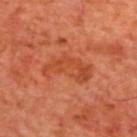Q: Is there a histopathology result?
A: imaged on a skin check; not biopsied
Q: What did automated image analysis measure?
A: an area of roughly 12 mm², a shape eccentricity near 0.9, and a symmetry-axis asymmetry near 0.4; a nevus-likeness score of about 0/100 and a detector confidence of about 100 out of 100 that the crop contains a lesion
Q: What is the lesion's diameter?
A: ~6 mm (longest diameter)
Q: Illumination type?
A: cross-polarized
Q: What is the imaging modality?
A: ~15 mm crop, total-body skin-cancer survey
Q: Where on the body is the lesion?
A: the mid back
Q: What are the patient's age and sex?
A: male, aged 68–72